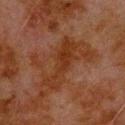The lesion was tiled from a total-body skin photograph and was not biopsied. A lesion tile, about 15 mm wide, cut from a 3D total-body photograph. This is a cross-polarized tile. An algorithmic analysis of the crop reported a lesion area of about 11 mm², an eccentricity of roughly 0.95, and a shape-asymmetry score of about 0.45 (0 = symmetric). It also reported a lesion color around L≈26 a*≈20 b*≈28 in CIELAB and about 6 CIELAB-L* units darker than the surrounding skin. The subject is a male approximately 80 years of age. The lesion is on the upper back. About 6.5 mm across.